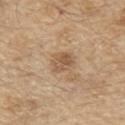The lesion was photographed on a routine skin check and not biopsied; there is no pathology result.
The total-body-photography lesion software estimated a border-irregularity index near 1.5/10, a color-variation rating of about 3.5/10, and peripheral color asymmetry of about 1.5. The software also gave a classifier nevus-likeness of about 50/100 and lesion-presence confidence of about 100/100.
About 3 mm across.
A 15 mm close-up extracted from a 3D total-body photography capture.
A male patient in their 70s.
The lesion is located on the front of the torso.
Imaged with white-light lighting.